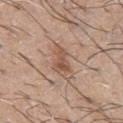biopsy_status: not biopsied; imaged during a skin examination
automated_metrics:
  area_mm2_approx: 6.0
  eccentricity: 0.8
  shape_asymmetry: 0.4
  cielab_L: 53
  cielab_a: 19
  cielab_b: 29
  vs_skin_darker_L: 9.0
  vs_skin_contrast_norm: 7.0
  lesion_detection_confidence_0_100: 100
site: front of the torso
image:
  source: total-body photography crop
  field_of_view_mm: 15
patient:
  sex: male
  age_approx: 65